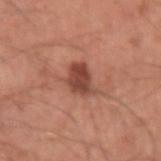acquisition: ~15 mm tile from a whole-body skin photo
illumination: white-light
site: the left upper arm
subject: male, about 60 years old
TBP lesion metrics: a lesion area of about 7.5 mm² and a shape-asymmetry score of about 0.3 (0 = symmetric); a mean CIELAB color near L≈46 a*≈25 b*≈28, about 13 CIELAB-L* units darker than the surrounding skin, and a normalized lesion–skin contrast near 9; an automated nevus-likeness rating near 75 out of 100 and a lesion-detection confidence of about 100/100
size: about 3.5 mm